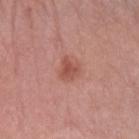{"biopsy_status": "not biopsied; imaged during a skin examination", "lesion_size": {"long_diameter_mm_approx": 2.5}, "automated_metrics": {"nevus_likeness_0_100": 70, "lesion_detection_confidence_0_100": 100}, "site": "left arm", "patient": {"sex": "female", "age_approx": 65}, "lighting": "white-light", "image": {"source": "total-body photography crop", "field_of_view_mm": 15}}